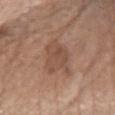Case summary:
- biopsy status · no biopsy performed (imaged during a skin exam)
- diameter · ~5 mm (longest diameter)
- subject · female, aged 63–67
- imaging modality · ~15 mm crop, total-body skin-cancer survey
- site · the chest